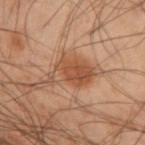The tile uses cross-polarized illumination.
The lesion is located on the left forearm.
A lesion tile, about 15 mm wide, cut from a 3D total-body photograph.
The subject is a male in their 50s.
Automated image analysis of the tile measured a lesion area of about 11 mm² and an outline eccentricity of about 0.85 (0 = round, 1 = elongated). The analysis additionally found roughly 8 lightness units darker than nearby skin and a normalized border contrast of about 7.5. And it measured border irregularity of about 4 on a 0–10 scale, a within-lesion color-variation index near 3.5/10, and peripheral color asymmetry of about 1. And it measured a nevus-likeness score of about 75/100 and a lesion-detection confidence of about 100/100.
Longest diameter approximately 5.5 mm.